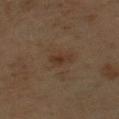follow-up: total-body-photography surveillance lesion; no biopsy
image source: ~15 mm crop, total-body skin-cancer survey
subject: male, aged approximately 60
site: the left upper arm
size: ~2.5 mm (longest diameter)